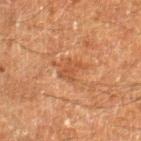TBP lesion metrics: internal color variation of about 1 on a 0–10 scale; a detector confidence of about 100 out of 100 that the crop contains a lesion | illumination: cross-polarized illumination | image: total-body-photography crop, ~15 mm field of view | body site: the right lower leg | subject: male, aged 58 to 62.